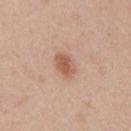Case summary:
- biopsy status — total-body-photography surveillance lesion; no biopsy
- anatomic site — the mid back
- image source — ~15 mm tile from a whole-body skin photo
- subject — male, aged 33 to 37